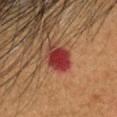Recorded during total-body skin imaging; not selected for excision or biopsy.
From the head or neck.
A 15 mm crop from a total-body photograph taken for skin-cancer surveillance.
About 3 mm across.
The tile uses cross-polarized illumination.
The total-body-photography lesion software estimated a mean CIELAB color near L≈33 a*≈31 b*≈25, about 13 CIELAB-L* units darker than the surrounding skin, and a lesion-to-skin contrast of about 12 (normalized; higher = more distinct). The analysis additionally found a detector confidence of about 100 out of 100 that the crop contains a lesion.
A male subject, aged 53 to 57.